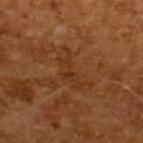Imaged during a routine full-body skin examination; the lesion was not biopsied and no histopathology is available. The recorded lesion diameter is about 5 mm. A 15 mm close-up tile from a total-body photography series done for melanoma screening. The lesion-visualizer software estimated an outline eccentricity of about 0.95 (0 = round, 1 = elongated) and a shape-asymmetry score of about 0.55 (0 = symmetric). The software also gave a lesion–skin lightness drop of about 5 and a normalized border contrast of about 5.5. The analysis additionally found a border-irregularity index near 9/10, a within-lesion color-variation index near 1/10, and peripheral color asymmetry of about 0.5. A male subject, aged 63–67.